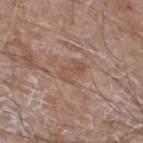  site: left lower leg
  image:
    source: total-body photography crop
    field_of_view_mm: 15
  patient:
    sex: male
    age_approx: 60
  automated_metrics:
    eccentricity: 0.8
    shape_asymmetry: 0.35
    cielab_L: 51
    cielab_a: 18
    cielab_b: 27
    vs_skin_darker_L: 6.0
    border_irregularity_0_10: 4.5
    color_variation_0_10: 2.0
    peripheral_color_asymmetry: 1.0
  lighting: white-light
  lesion_size:
    long_diameter_mm_approx: 3.0